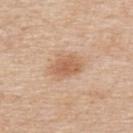Captured during whole-body skin photography for melanoma surveillance; the lesion was not biopsied. Imaged with white-light lighting. This image is a 15 mm lesion crop taken from a total-body photograph. Located on the upper back. A male subject, about 60 years old. An algorithmic analysis of the crop reported radial color variation of about 0.5. Measured at roughly 4 mm in maximum diameter.